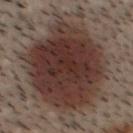The lesion was tiled from a total-body skin photograph and was not biopsied.
A roughly 15 mm field-of-view crop from a total-body skin photograph.
An algorithmic analysis of the crop reported a mean CIELAB color near L≈27 a*≈13 b*≈17, about 10 CIELAB-L* units darker than the surrounding skin, and a lesion-to-skin contrast of about 10.5 (normalized; higher = more distinct). The analysis additionally found border irregularity of about 3.5 on a 0–10 scale, internal color variation of about 4.5 on a 0–10 scale, and radial color variation of about 1. The software also gave a detector confidence of about 100 out of 100 that the crop contains a lesion.
Approximately 13.5 mm at its widest.
This is a cross-polarized tile.
A male subject aged around 30.
On the head or neck.